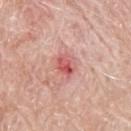Part of a total-body skin-imaging series; this lesion was reviewed on a skin check and was not flagged for biopsy. A male patient, about 75 years old. This image is a 15 mm lesion crop taken from a total-body photograph. The lesion is on the upper back.